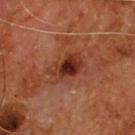biopsy status: catalogued during a skin exam; not biopsied | patient: male, about 55 years old | imaging modality: ~15 mm crop, total-body skin-cancer survey | anatomic site: the chest.